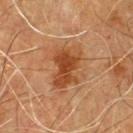Findings:
- biopsy status · no biopsy performed (imaged during a skin exam)
- subject · male, aged around 60
- image-analysis metrics · a lesion area of about 14 mm² and a shape eccentricity near 0.75; a border-irregularity rating of about 3.5/10, a color-variation rating of about 5/10, and radial color variation of about 1.5
- acquisition · 15 mm crop, total-body photography
- site · the chest
- tile lighting · cross-polarized
- size · ≈5 mm